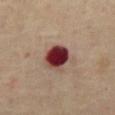Part of a total-body skin-imaging series; this lesion was reviewed on a skin check and was not flagged for biopsy. A close-up tile cropped from a whole-body skin photograph, about 15 mm across. The lesion is on the abdomen. The patient is a male approximately 70 years of age.